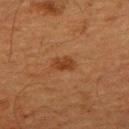Background:
A male patient, aged 63 to 67. Automated image analysis of the tile measured radial color variation of about 0.5. This is a cross-polarized tile. This image is a 15 mm lesion crop taken from a total-body photograph. About 2.5 mm across. From the upper back.